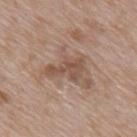Acquisition and patient details: A male subject, aged 63–67. The lesion is on the upper back. A 15 mm close-up extracted from a 3D total-body photography capture. Automated image analysis of the tile measured a mean CIELAB color near L≈51 a*≈17 b*≈27 and a lesion–skin lightness drop of about 8. It also reported a border-irregularity rating of about 5.5/10 and a color-variation rating of about 2.5/10. The recorded lesion diameter is about 4.5 mm.